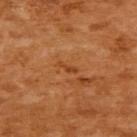{"biopsy_status": "not biopsied; imaged during a skin examination", "image": {"source": "total-body photography crop", "field_of_view_mm": 15}, "patient": {"sex": "female", "age_approx": 55}, "lesion_size": {"long_diameter_mm_approx": 2.5}, "site": "upper back", "automated_metrics": {"area_mm2_approx": 2.0, "eccentricity": 0.95, "shape_asymmetry": 0.5, "border_irregularity_0_10": 5.5}, "lighting": "cross-polarized"}